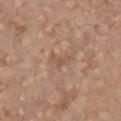Acquisition and patient details: A 15 mm close-up tile from a total-body photography series done for melanoma screening. A male patient, aged around 70. The lesion is on the front of the torso.